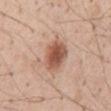Assessment:
Imaged during a routine full-body skin examination; the lesion was not biopsied and no histopathology is available.
Background:
A 15 mm crop from a total-body photograph taken for skin-cancer surveillance. A male patient in their mid- to late 50s. Measured at roughly 4 mm in maximum diameter. The total-body-photography lesion software estimated an average lesion color of about L≈55 a*≈22 b*≈30 (CIELAB) and roughly 14 lightness units darker than nearby skin. The software also gave a border-irregularity index near 1.5/10, a color-variation rating of about 4.5/10, and a peripheral color-asymmetry measure near 1. On the abdomen.